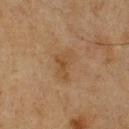Impression: No biopsy was performed on this lesion — it was imaged during a full skin examination and was not determined to be concerning. Image and clinical context: About 4 mm across. A male patient, in their mid-70s. A 15 mm crop from a total-body photograph taken for skin-cancer surveillance. On the front of the torso.